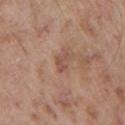<record>
<biopsy_status>not biopsied; imaged during a skin examination</biopsy_status>
<lighting>white-light</lighting>
<patient>
  <sex>male</sex>
  <age_approx>55</age_approx>
</patient>
<site>chest</site>
<lesion_size>
  <long_diameter_mm_approx>2.5</long_diameter_mm_approx>
</lesion_size>
<image>
  <source>total-body photography crop</source>
  <field_of_view_mm>15</field_of_view_mm>
</image>
</record>